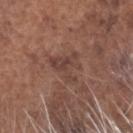location = the head or neck; patient = male, aged 73 to 77; acquisition = 15 mm crop, total-body photography.